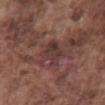Clinical impression: No biopsy was performed on this lesion — it was imaged during a full skin examination and was not determined to be concerning. Clinical summary: From the abdomen. A close-up tile cropped from a whole-body skin photograph, about 15 mm across. Captured under white-light illumination. The lesion-visualizer software estimated an area of roughly 7 mm² and a symmetry-axis asymmetry near 0.25. The analysis additionally found a lesion color around L≈35 a*≈19 b*≈19 in CIELAB, about 7 CIELAB-L* units darker than the surrounding skin, and a normalized border contrast of about 7. The software also gave internal color variation of about 4 on a 0–10 scale and peripheral color asymmetry of about 1.5. And it measured a nevus-likeness score of about 0/100 and a detector confidence of about 70 out of 100 that the crop contains a lesion. About 3.5 mm across. A male patient aged 73–77.